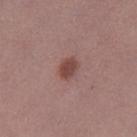Clinical impression: No biopsy was performed on this lesion — it was imaged during a full skin examination and was not determined to be concerning. Acquisition and patient details: A 15 mm close-up extracted from a 3D total-body photography capture. The lesion is on the leg. A female subject, roughly 50 years of age.